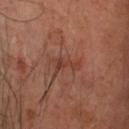Recorded during total-body skin imaging; not selected for excision or biopsy.
The lesion is located on the head or neck.
A close-up tile cropped from a whole-body skin photograph, about 15 mm across.
A male patient, aged around 50.